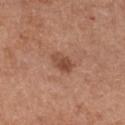Background:
The patient is a female in their 50s. Approximately 3 mm at its widest. The lesion is on the front of the torso. The tile uses white-light illumination. A region of skin cropped from a whole-body photographic capture, roughly 15 mm wide.